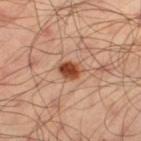Captured during whole-body skin photography for melanoma surveillance; the lesion was not biopsied.
A male subject aged 53–57.
A 15 mm close-up tile from a total-body photography series done for melanoma screening.
The lesion-visualizer software estimated an area of roughly 5.5 mm² and a shape-asymmetry score of about 0.2 (0 = symmetric). The software also gave roughly 15 lightness units darker than nearby skin and a normalized lesion–skin contrast near 11. And it measured a color-variation rating of about 5.5/10 and a peripheral color-asymmetry measure near 2. It also reported a classifier nevus-likeness of about 100/100 and a lesion-detection confidence of about 100/100.
The tile uses cross-polarized illumination.
From the leg.